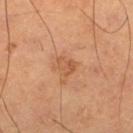Impression: No biopsy was performed on this lesion — it was imaged during a full skin examination and was not determined to be concerning.